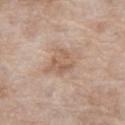| field | value |
|---|---|
| workup | catalogued during a skin exam; not biopsied |
| lesion diameter | ~4.5 mm (longest diameter) |
| subject | female, about 75 years old |
| site | the right thigh |
| image-analysis metrics | an average lesion color of about L≈60 a*≈17 b*≈29 (CIELAB), roughly 8 lightness units darker than nearby skin, and a normalized border contrast of about 6; a border-irregularity index near 3/10, a within-lesion color-variation index near 3.5/10, and peripheral color asymmetry of about 1.5 |
| imaging modality | ~15 mm tile from a whole-body skin photo |